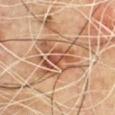<record>
<lesion_size>
  <long_diameter_mm_approx>8.0</long_diameter_mm_approx>
</lesion_size>
<lighting>cross-polarized</lighting>
<patient>
  <sex>male</sex>
  <age_approx>60</age_approx>
</patient>
<image>
  <source>total-body photography crop</source>
  <field_of_view_mm>15</field_of_view_mm>
</image>
<site>upper back</site>
</record>